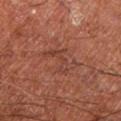Q: Is there a histopathology result?
A: no biopsy performed (imaged during a skin exam)
Q: How large is the lesion?
A: ≈4.5 mm
Q: What are the patient's age and sex?
A: male, in their mid-60s
Q: What kind of image is this?
A: total-body-photography crop, ~15 mm field of view
Q: Illumination type?
A: cross-polarized illumination
Q: Where on the body is the lesion?
A: the left lower leg